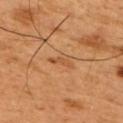{
  "biopsy_status": "not biopsied; imaged during a skin examination",
  "image": {
    "source": "total-body photography crop",
    "field_of_view_mm": 15
  },
  "lighting": "cross-polarized",
  "patient": {
    "sex": "male",
    "age_approx": 50
  },
  "site": "upper back"
}